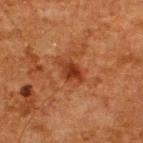Clinical impression:
Recorded during total-body skin imaging; not selected for excision or biopsy.
Clinical summary:
The lesion is located on the back. A male subject, aged 58–62. A 15 mm close-up tile from a total-body photography series done for melanoma screening. The lesion-visualizer software estimated a lesion area of about 4 mm², a shape eccentricity near 0.7, and a shape-asymmetry score of about 0.35 (0 = symmetric). And it measured about 9 CIELAB-L* units darker than the surrounding skin and a normalized border contrast of about 8.5. The software also gave a nevus-likeness score of about 50/100 and a detector confidence of about 100 out of 100 that the crop contains a lesion. The tile uses cross-polarized illumination. About 3 mm across.